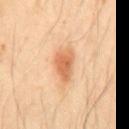{
  "biopsy_status": "not biopsied; imaged during a skin examination",
  "site": "mid back",
  "lesion_size": {
    "long_diameter_mm_approx": 4.5
  },
  "patient": {
    "sex": "male",
    "age_approx": 50
  },
  "lighting": "cross-polarized",
  "automated_metrics": {
    "nevus_likeness_0_100": 95,
    "lesion_detection_confidence_0_100": 100
  },
  "image": {
    "source": "total-body photography crop",
    "field_of_view_mm": 15
  }
}